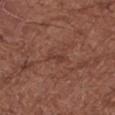Recorded during total-body skin imaging; not selected for excision or biopsy. Automated image analysis of the tile measured a footprint of about 2.5 mm² and a symmetry-axis asymmetry near 0.4. And it measured an average lesion color of about L≈38 a*≈23 b*≈25 (CIELAB), roughly 6 lightness units darker than nearby skin, and a normalized lesion–skin contrast near 5.5. It also reported a nevus-likeness score of about 0/100 and a detector confidence of about 100 out of 100 that the crop contains a lesion. Captured under white-light illumination. From the right forearm. A female patient aged 78–82. Cropped from a total-body skin-imaging series; the visible field is about 15 mm. About 3 mm across.